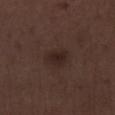Case summary:
* workup: total-body-photography surveillance lesion; no biopsy
* site: the left lower leg
* TBP lesion metrics: an average lesion color of about L≈22 a*≈14 b*≈17 (CIELAB)
* diameter: about 2.5 mm
* subject: male, aged around 70
* lighting: white-light illumination
* image source: ~15 mm crop, total-body skin-cancer survey